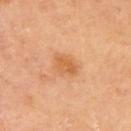diameter: ~3 mm (longest diameter)
imaging modality: ~15 mm tile from a whole-body skin photo
subject: female, aged around 65
lighting: cross-polarized illumination
body site: the left upper arm